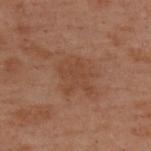Findings:
* workup · catalogued during a skin exam; not biopsied
* lighting · cross-polarized
* imaging modality · ~15 mm crop, total-body skin-cancer survey
* anatomic site · the upper back
* TBP lesion metrics · a lesion area of about 11 mm² and an eccentricity of roughly 0.6; a lesion–skin lightness drop of about 5 and a lesion-to-skin contrast of about 5 (normalized; higher = more distinct)
* subject · female, in their mid-50s